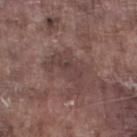{"site": "left lower leg", "automated_metrics": {"area_mm2_approx": 12.0, "eccentricity": 0.75, "shape_asymmetry": 0.45, "cielab_L": 41, "cielab_a": 17, "cielab_b": 18, "vs_skin_darker_L": 7.0, "vs_skin_contrast_norm": 5.5, "nevus_likeness_0_100": 0, "lesion_detection_confidence_0_100": 65}, "patient": {"sex": "male", "age_approx": 75}, "lesion_size": {"long_diameter_mm_approx": 5.0}, "image": {"source": "total-body photography crop", "field_of_view_mm": 15}}